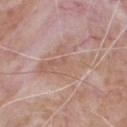follow-up — catalogued during a skin exam; not biopsied | acquisition — 15 mm crop, total-body photography | patient — male, aged 63 to 67 | tile lighting — white-light illumination | size — about 6 mm | automated lesion analysis — a lesion color around L≈59 a*≈18 b*≈26 in CIELAB, roughly 6 lightness units darker than nearby skin, and a lesion-to-skin contrast of about 5 (normalized; higher = more distinct); border irregularity of about 9.5 on a 0–10 scale, a within-lesion color-variation index near 3/10, and radial color variation of about 1 | body site — the chest.